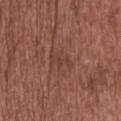| feature | finding |
|---|---|
| biopsy status | total-body-photography surveillance lesion; no biopsy |
| lighting | white-light |
| image source | ~15 mm crop, total-body skin-cancer survey |
| lesion size | ≈3 mm |
| patient | male, approximately 45 years of age |
| body site | the head or neck |
| automated lesion analysis | a lesion color around L≈40 a*≈22 b*≈25 in CIELAB and a normalized lesion–skin contrast near 5; radial color variation of about 0; a classifier nevus-likeness of about 0/100 and a detector confidence of about 65 out of 100 that the crop contains a lesion |